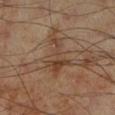follow-up: imaged on a skin check; not biopsied | image source: ~15 mm tile from a whole-body skin photo | tile lighting: cross-polarized | patient: male, about 70 years old | lesion size: ~5 mm (longest diameter) | site: the left lower leg.